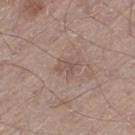<tbp_lesion>
<biopsy_status>not biopsied; imaged during a skin examination</biopsy_status>
<image>
  <source>total-body photography crop</source>
  <field_of_view_mm>15</field_of_view_mm>
</image>
<lesion_size>
  <long_diameter_mm_approx>3.0</long_diameter_mm_approx>
</lesion_size>
<site>left thigh</site>
<lighting>white-light</lighting>
<patient>
  <sex>male</sex>
  <age_approx>65</age_approx>
</patient>
</tbp_lesion>